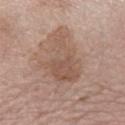Case summary:
• follow-up — imaged on a skin check; not biopsied
• location — the left forearm
• subject — female, roughly 65 years of age
• image source — total-body-photography crop, ~15 mm field of view